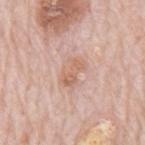Impression: The lesion was tiled from a total-body skin photograph and was not biopsied. Acquisition and patient details: Cropped from a total-body skin-imaging series; the visible field is about 15 mm. On the mid back. A male subject approximately 80 years of age.